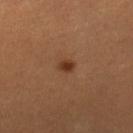{"biopsy_status": "not biopsied; imaged during a skin examination", "site": "right lower leg", "automated_metrics": {"area_mm2_approx": 3.0, "eccentricity": 0.55, "shape_asymmetry": 0.25, "cielab_L": 36, "cielab_a": 22, "cielab_b": 31, "vs_skin_darker_L": 9.0, "vs_skin_contrast_norm": 8.5}, "image": {"source": "total-body photography crop", "field_of_view_mm": 15}, "patient": {"sex": "female", "age_approx": 40}}